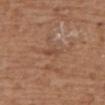Captured during whole-body skin photography for melanoma surveillance; the lesion was not biopsied. A 15 mm close-up tile from a total-body photography series done for melanoma screening. The patient is a female aged 73 to 77. The lesion is on the upper back.